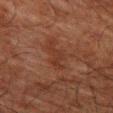{"image": {"source": "total-body photography crop", "field_of_view_mm": 15}, "patient": {"sex": "male", "age_approx": 80}, "site": "left thigh"}